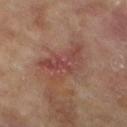notes = imaged on a skin check; not biopsied
site = the right lower leg
lesion diameter = about 5.5 mm
automated metrics = a lesion area of about 13 mm² and an eccentricity of roughly 0.75
tile lighting = cross-polarized
imaging modality = total-body-photography crop, ~15 mm field of view
patient = female, roughly 75 years of age